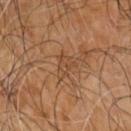Clinical impression: The lesion was tiled from a total-body skin photograph and was not biopsied. Background: Captured under cross-polarized illumination. A male subject approximately 60 years of age. A roughly 15 mm field-of-view crop from a total-body skin photograph. On the right arm.